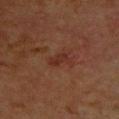Clinical impression:
The lesion was photographed on a routine skin check and not biopsied; there is no pathology result.
Clinical summary:
A 15 mm close-up extracted from a 3D total-body photography capture. On the upper back. Measured at roughly 3 mm in maximum diameter. Automated image analysis of the tile measured an area of roughly 4 mm² and an eccentricity of roughly 0.85. The software also gave a border-irregularity rating of about 3.5/10 and a color-variation rating of about 2/10. The analysis additionally found an automated nevus-likeness rating near 10 out of 100 and lesion-presence confidence of about 100/100. This is a cross-polarized tile. A female subject, about 80 years old.